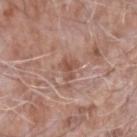follow-up=total-body-photography surveillance lesion; no biopsy
patient=male, in their mid- to late 70s
lesion diameter=~3 mm (longest diameter)
image=~15 mm crop, total-body skin-cancer survey
site=the right forearm